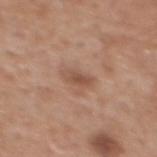<tbp_lesion>
<biopsy_status>not biopsied; imaged during a skin examination</biopsy_status>
<site>mid back</site>
<patient>
  <sex>male</sex>
  <age_approx>60</age_approx>
</patient>
<lighting>white-light</lighting>
<image>
  <source>total-body photography crop</source>
  <field_of_view_mm>15</field_of_view_mm>
</image>
</tbp_lesion>